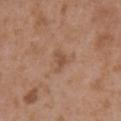<tbp_lesion>
<biopsy_status>not biopsied; imaged during a skin examination</biopsy_status>
<lesion_size>
  <long_diameter_mm_approx>3.0</long_diameter_mm_approx>
</lesion_size>
<site>right upper arm</site>
<patient>
  <sex>female</sex>
  <age_approx>35</age_approx>
</patient>
<lighting>white-light</lighting>
<image>
  <source>total-body photography crop</source>
  <field_of_view_mm>15</field_of_view_mm>
</image>
<automated_metrics>
  <border_irregularity_0_10>5.0</border_irregularity_0_10>
  <color_variation_0_10>1.0</color_variation_0_10>
</automated_metrics>
</tbp_lesion>